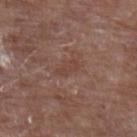{"site": "left forearm", "patient": {"sex": "male", "age_approx": 80}, "image": {"source": "total-body photography crop", "field_of_view_mm": 15}, "lighting": "white-light"}